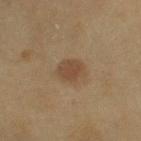biopsy_status: not biopsied; imaged during a skin examination
lesion_size:
  long_diameter_mm_approx: 3.0
lighting: cross-polarized
image:
  source: total-body photography crop
  field_of_view_mm: 15
patient:
  sex: female
  age_approx: 60
site: right upper arm